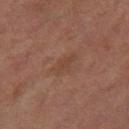Notes:
• workup · catalogued during a skin exam; not biopsied
• image · total-body-photography crop, ~15 mm field of view
• site · the right thigh
• patient · female, aged 58 to 62
• illumination · cross-polarized illumination